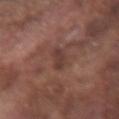follow-up: total-body-photography surveillance lesion; no biopsy
tile lighting: white-light illumination
location: the right forearm
subject: male, roughly 75 years of age
diameter: ~3 mm (longest diameter)
image source: ~15 mm tile from a whole-body skin photo